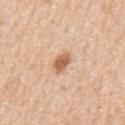Clinical impression: The lesion was photographed on a routine skin check and not biopsied; there is no pathology result. Image and clinical context: The patient is a male aged approximately 70. This is a white-light tile. The total-body-photography lesion software estimated an average lesion color of about L≈61 a*≈21 b*≈33 (CIELAB) and a normalized lesion–skin contrast near 8.5. The software also gave lesion-presence confidence of about 100/100. Approximately 3 mm at its widest. From the mid back. A close-up tile cropped from a whole-body skin photograph, about 15 mm across.